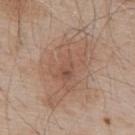No biopsy was performed on this lesion — it was imaged during a full skin examination and was not determined to be concerning. The lesion's longest dimension is about 9.5 mm. Automated image analysis of the tile measured an average lesion color of about L≈56 a*≈17 b*≈28 (CIELAB), a lesion–skin lightness drop of about 8, and a normalized border contrast of about 5.5. The analysis additionally found a border-irregularity rating of about 3.5/10, internal color variation of about 5 on a 0–10 scale, and peripheral color asymmetry of about 1.5. The software also gave an automated nevus-likeness rating near 5 out of 100 and a detector confidence of about 100 out of 100 that the crop contains a lesion. The lesion is located on the back. A male subject aged 53 to 57. A 15 mm crop from a total-body photograph taken for skin-cancer surveillance.